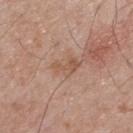The lesion was tiled from a total-body skin photograph and was not biopsied. A male patient, approximately 70 years of age. The lesion is on the upper back. A roughly 15 mm field-of-view crop from a total-body skin photograph.